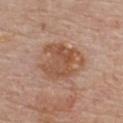Imaged during a routine full-body skin examination; the lesion was not biopsied and no histopathology is available.
The recorded lesion diameter is about 5.5 mm.
The lesion-visualizer software estimated an area of roughly 18 mm², an eccentricity of roughly 0.5, and a shape-asymmetry score of about 0.3 (0 = symmetric). The analysis additionally found lesion-presence confidence of about 100/100.
A male subject in their 60s.
From the chest.
A roughly 15 mm field-of-view crop from a total-body skin photograph.